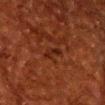notes: total-body-photography surveillance lesion; no biopsy | size: ≈3 mm | subject: male, roughly 60 years of age | imaging modality: total-body-photography crop, ~15 mm field of view | anatomic site: the head or neck.